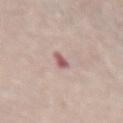Measured at roughly 2.5 mm in maximum diameter.
The patient is a female aged 63 to 67.
Captured under white-light illumination.
A lesion tile, about 15 mm wide, cut from a 3D total-body photograph.
Automated tile analysis of the lesion measured an automated nevus-likeness rating near 0 out of 100 and lesion-presence confidence of about 100/100.
The lesion is on the abdomen.